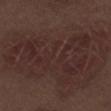Captured during whole-body skin photography for melanoma surveillance; the lesion was not biopsied.
An algorithmic analysis of the crop reported an area of roughly 125 mm² and an eccentricity of roughly 0.8. And it measured about 5 CIELAB-L* units darker than the surrounding skin and a normalized border contrast of about 5.5. It also reported a border-irregularity index near 4.5/10 and internal color variation of about 4 on a 0–10 scale. It also reported an automated nevus-likeness rating near 0 out of 100.
From the leg.
A roughly 15 mm field-of-view crop from a total-body skin photograph.
A male subject, aged 68–72.
Longest diameter approximately 17 mm.
Imaged with white-light lighting.